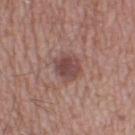biopsy status = total-body-photography surveillance lesion; no biopsy | tile lighting = white-light | body site = the right lower leg | subject = male, aged 53 to 57 | image = 15 mm crop, total-body photography.